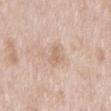Background: Cropped from a total-body skin-imaging series; the visible field is about 15 mm. A male subject, aged approximately 65. On the mid back. Automated image analysis of the tile measured an average lesion color of about L≈65 a*≈17 b*≈29 (CIELAB), about 7 CIELAB-L* units darker than the surrounding skin, and a normalized lesion–skin contrast near 5. The software also gave an automated nevus-likeness rating near 0 out of 100. Imaged with white-light lighting. The recorded lesion diameter is about 3 mm.